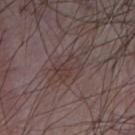Clinical impression:
This lesion was catalogued during total-body skin photography and was not selected for biopsy.
Acquisition and patient details:
Automated image analysis of the tile measured an area of roughly 6 mm² and a shape-asymmetry score of about 0.3 (0 = symmetric). The analysis additionally found an average lesion color of about L≈37 a*≈16 b*≈16 (CIELAB), about 6 CIELAB-L* units darker than the surrounding skin, and a normalized border contrast of about 5.5. It also reported a border-irregularity rating of about 4/10, a color-variation rating of about 4/10, and radial color variation of about 1.5. A 15 mm crop from a total-body photograph taken for skin-cancer surveillance. On the chest. The tile uses white-light illumination. A male subject in their mid-70s.